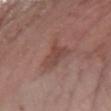{"biopsy_status": "not biopsied; imaged during a skin examination", "image": {"source": "total-body photography crop", "field_of_view_mm": 15}, "patient": {"sex": "female", "age_approx": "85+"}, "site": "left forearm"}